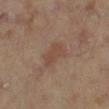Q: What is the imaging modality?
A: 15 mm crop, total-body photography
Q: What is the anatomic site?
A: the right lower leg
Q: How was the tile lit?
A: cross-polarized illumination
Q: Lesion size?
A: ~4 mm (longest diameter)
Q: Who is the patient?
A: male, aged 63 to 67
Q: Automated lesion metrics?
A: an area of roughly 7 mm², a shape eccentricity near 0.8, and a symmetry-axis asymmetry near 0.35; border irregularity of about 4 on a 0–10 scale and radial color variation of about 0.5